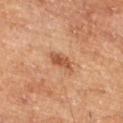No biopsy was performed on this lesion — it was imaged during a full skin examination and was not determined to be concerning.
A region of skin cropped from a whole-body photographic capture, roughly 15 mm wide.
The lesion is on the left lower leg.
A male patient roughly 60 years of age.